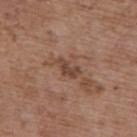Captured during whole-body skin photography for melanoma surveillance; the lesion was not biopsied. From the upper back. Cropped from a total-body skin-imaging series; the visible field is about 15 mm. The tile uses white-light illumination. A female patient aged around 65. The total-body-photography lesion software estimated a shape eccentricity near 0.85 and a shape-asymmetry score of about 0.4 (0 = symmetric). And it measured border irregularity of about 4.5 on a 0–10 scale and internal color variation of about 1.5 on a 0–10 scale. And it measured a nevus-likeness score of about 0/100 and a detector confidence of about 100 out of 100 that the crop contains a lesion.